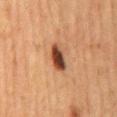Q: Is there a histopathology result?
A: catalogued during a skin exam; not biopsied
Q: What is the imaging modality?
A: total-body-photography crop, ~15 mm field of view
Q: Lesion size?
A: about 3.5 mm
Q: What lighting was used for the tile?
A: cross-polarized illumination
Q: Lesion location?
A: the mid back
Q: Patient demographics?
A: female, approximately 60 years of age
Q: What did automated image analysis measure?
A: a footprint of about 5.5 mm², a shape eccentricity near 0.85, and a symmetry-axis asymmetry near 0.2; a normalized border contrast of about 13.5; a lesion-detection confidence of about 100/100